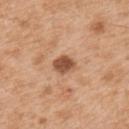Findings:
– follow-up · total-body-photography surveillance lesion; no biopsy
– subject · male, aged approximately 55
– lesion diameter · ~3 mm (longest diameter)
– illumination · white-light illumination
– image source · total-body-photography crop, ~15 mm field of view
– location · the upper back